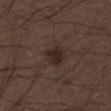Clinical impression:
The lesion was photographed on a routine skin check and not biopsied; there is no pathology result.
Clinical summary:
Imaged with white-light lighting. The lesion is located on the right thigh. The subject is a male aged 48–52. This image is a 15 mm lesion crop taken from a total-body photograph. About 3 mm across.